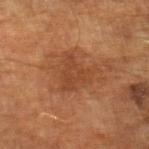biopsy_status: not biopsied; imaged during a skin examination
patient:
  sex: male
  age_approx: 65
lighting: cross-polarized
lesion_size:
  long_diameter_mm_approx: 4.0
image:
  source: total-body photography crop
  field_of_view_mm: 15
site: left leg